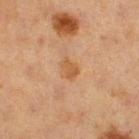Q: What are the patient's age and sex?
A: female, approximately 40 years of age
Q: Illumination type?
A: cross-polarized
Q: What is the imaging modality?
A: total-body-photography crop, ~15 mm field of view
Q: How large is the lesion?
A: about 2.5 mm
Q: Lesion location?
A: the right thigh